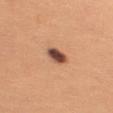body site: the right upper arm | acquisition: total-body-photography crop, ~15 mm field of view | subject: female, aged around 25 | TBP lesion metrics: a lesion color around L≈49 a*≈23 b*≈29 in CIELAB, roughly 19 lightness units darker than nearby skin, and a normalized border contrast of about 13; a border-irregularity index near 2/10, a within-lesion color-variation index near 5/10, and radial color variation of about 1.5; a nevus-likeness score of about 95/100 and lesion-presence confidence of about 100/100 | lighting: white-light illumination | diameter: ≈3 mm.